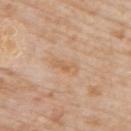{"biopsy_status": "not biopsied; imaged during a skin examination", "image": {"source": "total-body photography crop", "field_of_view_mm": 15}, "lighting": "white-light", "patient": {"sex": "male", "age_approx": 75}, "site": "arm"}